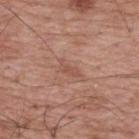Imaged during a routine full-body skin examination; the lesion was not biopsied and no histopathology is available. The subject is a male about 70 years old. Captured under white-light illumination. A 15 mm close-up extracted from a 3D total-body photography capture. Longest diameter approximately 3 mm. The total-body-photography lesion software estimated a lesion area of about 2.5 mm², an outline eccentricity of about 0.9 (0 = round, 1 = elongated), and a symmetry-axis asymmetry near 0.4. It also reported a mean CIELAB color near L≈52 a*≈22 b*≈29 and a lesion-to-skin contrast of about 5 (normalized; higher = more distinct). From the upper back.